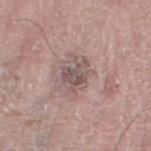Clinical impression:
Captured during whole-body skin photography for melanoma surveillance; the lesion was not biopsied.
Acquisition and patient details:
The lesion is located on the leg. The patient is a male about 75 years old. About 3.5 mm across. Cropped from a total-body skin-imaging series; the visible field is about 15 mm.